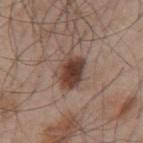No biopsy was performed on this lesion — it was imaged during a full skin examination and was not determined to be concerning. On the back. A male patient, about 55 years old. The recorded lesion diameter is about 4 mm. Imaged with white-light lighting. A close-up tile cropped from a whole-body skin photograph, about 15 mm across.